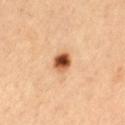workup = catalogued during a skin exam; not biopsied
patient = male, approximately 65 years of age
site = the left thigh
imaging modality = 15 mm crop, total-body photography
illumination = cross-polarized
automated metrics = a lesion area of about 4.5 mm², an eccentricity of roughly 0.35, and two-axis asymmetry of about 0.15; a lesion color around L≈55 a*≈26 b*≈38 in CIELAB and a normalized lesion–skin contrast near 12.5; border irregularity of about 1 on a 0–10 scale, a within-lesion color-variation index near 9/10, and a peripheral color-asymmetry measure near 4; lesion-presence confidence of about 100/100
size = ≈2.5 mm